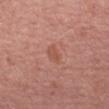{"biopsy_status": "not biopsied; imaged during a skin examination", "image": {"source": "total-body photography crop", "field_of_view_mm": 15}, "site": "arm", "patient": {"sex": "female", "age_approx": 60}, "lighting": "white-light"}